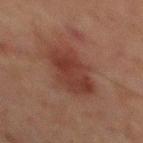Imaged during a routine full-body skin examination; the lesion was not biopsied and no histopathology is available.
The recorded lesion diameter is about 6 mm.
The patient is a male roughly 65 years of age.
Cropped from a whole-body photographic skin survey; the tile spans about 15 mm.
Located on the abdomen.
The tile uses cross-polarized illumination.